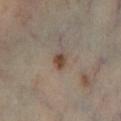No biopsy was performed on this lesion — it was imaged during a full skin examination and was not determined to be concerning.
Located on the left lower leg.
A male patient, approximately 55 years of age.
A 15 mm close-up extracted from a 3D total-body photography capture.